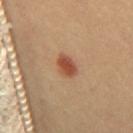follow-up — total-body-photography surveillance lesion; no biopsy | body site — the mid back | size — ≈3 mm | image — ~15 mm crop, total-body skin-cancer survey | subject — female, about 30 years old | automated metrics — a mean CIELAB color near L≈50 a*≈25 b*≈33; a classifier nevus-likeness of about 100/100.